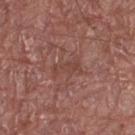follow-up = imaged on a skin check; not biopsied | TBP lesion metrics = a footprint of about 4.5 mm², an eccentricity of roughly 0.85, and two-axis asymmetry of about 0.45; border irregularity of about 5.5 on a 0–10 scale, internal color variation of about 2 on a 0–10 scale, and peripheral color asymmetry of about 0.5 | image = ~15 mm tile from a whole-body skin photo | patient = male, about 75 years old | tile lighting = white-light illumination | location = the right thigh | lesion size = ≈3.5 mm.